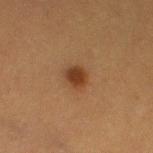| key | value |
|---|---|
| workup | total-body-photography surveillance lesion; no biopsy |
| image source | total-body-photography crop, ~15 mm field of view |
| size | about 2.5 mm |
| body site | the left thigh |
| tile lighting | cross-polarized |
| subject | female, aged 38–42 |